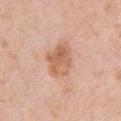Part of a total-body skin-imaging series; this lesion was reviewed on a skin check and was not flagged for biopsy.
Located on the left upper arm.
A male patient in their 60s.
Approximately 4 mm at its widest.
Cropped from a whole-body photographic skin survey; the tile spans about 15 mm.
The tile uses white-light illumination.